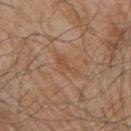The lesion was tiled from a total-body skin photograph and was not biopsied.
Captured under white-light illumination.
A male subject, aged 78–82.
This image is a 15 mm lesion crop taken from a total-body photograph.
Approximately 3 mm at its widest.
The lesion is located on the right upper arm.